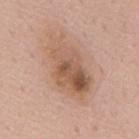Impression: The lesion was tiled from a total-body skin photograph and was not biopsied. Clinical summary: A 15 mm crop from a total-body photograph taken for skin-cancer surveillance. The lesion is on the mid back. The lesion's longest dimension is about 7.5 mm. Imaged with white-light lighting. A male patient, aged 53 to 57. The lesion-visualizer software estimated a lesion area of about 21 mm², an eccentricity of roughly 0.85, and two-axis asymmetry of about 0.2. And it measured a border-irregularity index near 3.5/10, internal color variation of about 8.5 on a 0–10 scale, and radial color variation of about 3.5.